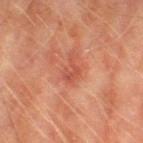Impression:
No biopsy was performed on this lesion — it was imaged during a full skin examination and was not determined to be concerning.
Image and clinical context:
A close-up tile cropped from a whole-body skin photograph, about 15 mm across. The patient is a male about 75 years old. On the leg.